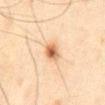Assessment:
This lesion was catalogued during total-body skin photography and was not selected for biopsy.
Image and clinical context:
Cropped from a whole-body photographic skin survey; the tile spans about 15 mm. Automated tile analysis of the lesion measured roughly 13 lightness units darker than nearby skin and a normalized lesion–skin contrast near 9.5. The software also gave a within-lesion color-variation index near 5.5/10 and peripheral color asymmetry of about 1.5. And it measured an automated nevus-likeness rating near 95 out of 100 and a lesion-detection confidence of about 100/100. The patient is a male aged 43 to 47. About 3 mm across. Located on the abdomen.